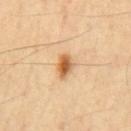Captured under cross-polarized illumination.
Cropped from a total-body skin-imaging series; the visible field is about 15 mm.
An algorithmic analysis of the crop reported a mean CIELAB color near L≈58 a*≈22 b*≈41 and a lesion-to-skin contrast of about 10 (normalized; higher = more distinct). The software also gave border irregularity of about 2 on a 0–10 scale, internal color variation of about 4.5 on a 0–10 scale, and peripheral color asymmetry of about 1.5.
The patient is a male about 60 years old.
The lesion is located on the chest.